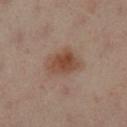notes — total-body-photography surveillance lesion; no biopsy
acquisition — 15 mm crop, total-body photography
patient — female, approximately 40 years of age
site — the right leg
lesion size — about 3.5 mm
lighting — cross-polarized illumination